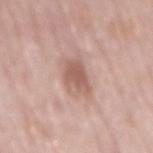Assessment:
Captured during whole-body skin photography for melanoma surveillance; the lesion was not biopsied.
Image and clinical context:
A region of skin cropped from a whole-body photographic capture, roughly 15 mm wide. Measured at roughly 4 mm in maximum diameter. The tile uses white-light illumination. On the mid back. A male subject roughly 75 years of age.